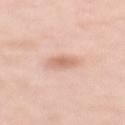This lesion was catalogued during total-body skin photography and was not selected for biopsy.